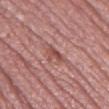Assessment: Imaged during a routine full-body skin examination; the lesion was not biopsied and no histopathology is available. Image and clinical context: Approximately 2.5 mm at its widest. This is a white-light tile. A 15 mm close-up tile from a total-body photography series done for melanoma screening. The lesion is located on the leg. The patient is a female aged 53–57.